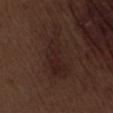{
  "biopsy_status": "not biopsied; imaged during a skin examination",
  "image": {
    "source": "total-body photography crop",
    "field_of_view_mm": 15
  },
  "site": "back",
  "automated_metrics": {
    "cielab_L": 22,
    "cielab_a": 16,
    "cielab_b": 18,
    "vs_skin_contrast_norm": 5.5,
    "nevus_likeness_0_100": 5
  },
  "lesion_size": {
    "long_diameter_mm_approx": 7.5
  },
  "patient": {
    "sex": "male",
    "age_approx": 70
  },
  "lighting": "white-light"
}